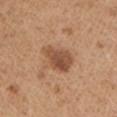Cropped from a whole-body photographic skin survey; the tile spans about 15 mm. Located on the left upper arm. A male subject, roughly 65 years of age.